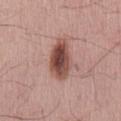A male subject in their mid-60s.
Measured at roughly 5 mm in maximum diameter.
Located on the mid back.
Automated tile analysis of the lesion measured a footprint of about 12 mm², an outline eccentricity of about 0.8 (0 = round, 1 = elongated), and a shape-asymmetry score of about 0.2 (0 = symmetric). And it measured about 16 CIELAB-L* units darker than the surrounding skin.
Cropped from a total-body skin-imaging series; the visible field is about 15 mm.
Captured under white-light illumination.